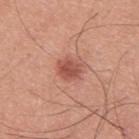illumination=white-light | patient=male, aged 28–32 | size=about 3 mm | acquisition=15 mm crop, total-body photography | location=the upper back | image-analysis metrics=a border-irregularity index near 2/10 and radial color variation of about 1; an automated nevus-likeness rating near 90 out of 100 and a detector confidence of about 100 out of 100 that the crop contains a lesion.